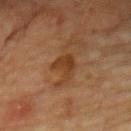Captured during whole-body skin photography for melanoma surveillance; the lesion was not biopsied.
Automated tile analysis of the lesion measured a footprint of about 5.5 mm², an outline eccentricity of about 0.7 (0 = round, 1 = elongated), and two-axis asymmetry of about 0.45. The software also gave roughly 8 lightness units darker than nearby skin and a normalized border contrast of about 8. It also reported a border-irregularity rating of about 4.5/10 and a within-lesion color-variation index near 2/10.
The patient is a male in their mid-80s or older.
The lesion is on the chest.
This is a cross-polarized tile.
Longest diameter approximately 3.5 mm.
A lesion tile, about 15 mm wide, cut from a 3D total-body photograph.